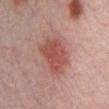Captured during whole-body skin photography for melanoma surveillance; the lesion was not biopsied. This image is a 15 mm lesion crop taken from a total-body photograph. About 5 mm across. Imaged with white-light lighting. The subject is a male in their 30s.